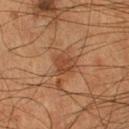biopsy status: imaged on a skin check; not biopsied
body site: the right lower leg
image source: ~15 mm tile from a whole-body skin photo
illumination: cross-polarized
patient: male, aged around 55
lesion size: about 3 mm